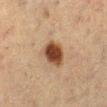follow-up = no biopsy performed (imaged during a skin exam)
diameter = ~3.5 mm (longest diameter)
site = the left lower leg
patient = female, aged 53–57
illumination = cross-polarized illumination
imaging modality = 15 mm crop, total-body photography